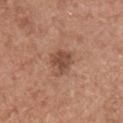Clinical impression:
The lesion was photographed on a routine skin check and not biopsied; there is no pathology result.
Background:
Cropped from a total-body skin-imaging series; the visible field is about 15 mm. The lesion is on the chest. Imaged with white-light lighting. A male subject, aged approximately 75.